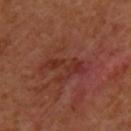No biopsy was performed on this lesion — it was imaged during a full skin examination and was not determined to be concerning. A female patient, aged approximately 40. A lesion tile, about 15 mm wide, cut from a 3D total-body photograph. The lesion is located on the left upper arm.